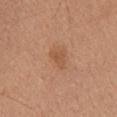Impression: The lesion was photographed on a routine skin check and not biopsied; there is no pathology result. Context: The lesion is on the back. A female patient, aged 43 to 47. A 15 mm close-up extracted from a 3D total-body photography capture.